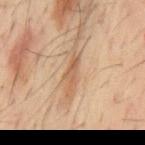The lesion was photographed on a routine skin check and not biopsied; there is no pathology result.
A male patient aged approximately 60.
The lesion is located on the mid back.
A lesion tile, about 15 mm wide, cut from a 3D total-body photograph.
The total-body-photography lesion software estimated a lesion color around L≈54 a*≈19 b*≈33 in CIELAB, roughly 10 lightness units darker than nearby skin, and a normalized border contrast of about 7. It also reported a border-irregularity index near 5.5/10 and peripheral color asymmetry of about 0.5. And it measured a nevus-likeness score of about 15/100 and a detector confidence of about 55 out of 100 that the crop contains a lesion.